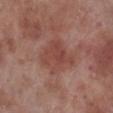Case summary:
- biopsy status — catalogued during a skin exam; not biopsied
- lesion diameter — ~4.5 mm (longest diameter)
- body site — the right lower leg
- imaging modality — ~15 mm crop, total-body skin-cancer survey
- image-analysis metrics — a lesion area of about 13 mm² and a symmetry-axis asymmetry near 0.3; a border-irregularity index near 3.5/10, internal color variation of about 3.5 on a 0–10 scale, and radial color variation of about 1
- subject — male, roughly 70 years of age
- lighting — white-light illumination